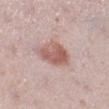| field | value |
|---|---|
| workup | no biopsy performed (imaged during a skin exam) |
| acquisition | ~15 mm tile from a whole-body skin photo |
| anatomic site | the leg |
| subject | female, aged approximately 40 |